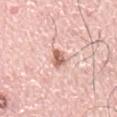Assessment: No biopsy was performed on this lesion — it was imaged during a full skin examination and was not determined to be concerning. Background: Located on the back. The subject is a male in their mid-70s. Approximately 3 mm at its widest. This image is a 15 mm lesion crop taken from a total-body photograph.